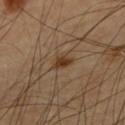Findings:
– notes · total-body-photography surveillance lesion; no biopsy
– site · the upper back
– tile lighting · cross-polarized illumination
– acquisition · total-body-photography crop, ~15 mm field of view
– TBP lesion metrics · an area of roughly 3.5 mm² and an eccentricity of roughly 0.7
– patient · male, roughly 65 years of age
– lesion diameter · ≈2.5 mm